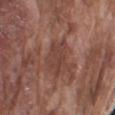imaging modality: 15 mm crop, total-body photography
illumination: white-light
automated lesion analysis: an area of roughly 6.5 mm², an outline eccentricity of about 0.8 (0 = round, 1 = elongated), and a shape-asymmetry score of about 0.45 (0 = symmetric); a border-irregularity index near 6.5/10, internal color variation of about 2 on a 0–10 scale, and a peripheral color-asymmetry measure near 0.5; a nevus-likeness score of about 0/100 and a detector confidence of about 80 out of 100 that the crop contains a lesion
lesion diameter: ~4 mm (longest diameter)
site: the right upper arm
patient: male, roughly 75 years of age